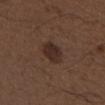Recorded during total-body skin imaging; not selected for excision or biopsy. A male patient, aged 48–52. Automated tile analysis of the lesion measured a footprint of about 7 mm² and a shape-asymmetry score of about 0.15 (0 = symmetric). The software also gave a mean CIELAB color near L≈28 a*≈16 b*≈21 and a lesion-to-skin contrast of about 8.5 (normalized; higher = more distinct). It also reported an automated nevus-likeness rating near 65 out of 100 and a detector confidence of about 100 out of 100 that the crop contains a lesion. A lesion tile, about 15 mm wide, cut from a 3D total-body photograph. Imaged with white-light lighting. On the right upper arm. The recorded lesion diameter is about 3.5 mm.